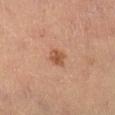Q: Was this lesion biopsied?
A: no biopsy performed (imaged during a skin exam)
Q: What is the imaging modality?
A: ~15 mm tile from a whole-body skin photo
Q: What are the patient's age and sex?
A: male, aged around 60
Q: What is the anatomic site?
A: the leg
Q: Automated lesion metrics?
A: a footprint of about 4 mm² and a shape eccentricity near 0.45; a border-irregularity rating of about 2/10 and a within-lesion color-variation index near 2/10
Q: What lighting was used for the tile?
A: cross-polarized illumination